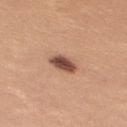Recorded during total-body skin imaging; not selected for excision or biopsy.
The lesion is on the arm.
A roughly 15 mm field-of-view crop from a total-body skin photograph.
Measured at roughly 3.5 mm in maximum diameter.
A female subject approximately 25 years of age.
The tile uses white-light illumination.